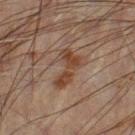{"biopsy_status": "not biopsied; imaged during a skin examination", "image": {"source": "total-body photography crop", "field_of_view_mm": 15}, "site": "left leg", "patient": {"sex": "male", "age_approx": 60}, "lesion_size": {"long_diameter_mm_approx": 5.0}}